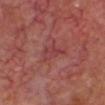The lesion was tiled from a total-body skin photograph and was not biopsied. From the head or neck. The subject is a male aged around 65. Captured under cross-polarized illumination. Cropped from a total-body skin-imaging series; the visible field is about 15 mm.